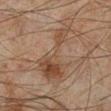Clinical impression:
This lesion was catalogued during total-body skin photography and was not selected for biopsy.
Background:
Captured under cross-polarized illumination. Measured at roughly 8 mm in maximum diameter. The lesion is on the left lower leg. The total-body-photography lesion software estimated a lesion area of about 18 mm², a shape eccentricity near 0.9, and two-axis asymmetry of about 0.75. It also reported a lesion color around L≈37 a*≈14 b*≈24 in CIELAB and about 7 CIELAB-L* units darker than the surrounding skin. It also reported a border-irregularity rating of about 10/10, internal color variation of about 4.5 on a 0–10 scale, and radial color variation of about 1.5. A 15 mm close-up extracted from a 3D total-body photography capture. A male patient, in their mid-40s.